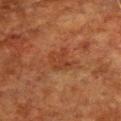Recorded during total-body skin imaging; not selected for excision or biopsy.
A 15 mm close-up tile from a total-body photography series done for melanoma screening.
From the chest.
The patient is a male aged 78–82.